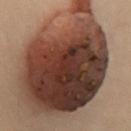Q: Was this lesion biopsied?
A: total-body-photography surveillance lesion; no biopsy
Q: What lighting was used for the tile?
A: cross-polarized
Q: Where on the body is the lesion?
A: the abdomen
Q: Automated lesion metrics?
A: an average lesion color of about L≈32 a*≈17 b*≈22 (CIELAB), about 19 CIELAB-L* units darker than the surrounding skin, and a normalized lesion–skin contrast near 16; a border-irregularity rating of about 3.5/10, a color-variation rating of about 9/10, and a peripheral color-asymmetry measure near 2.5
Q: What kind of image is this?
A: total-body-photography crop, ~15 mm field of view
Q: What is the lesion's diameter?
A: ≈15 mm
Q: Patient demographics?
A: female, aged approximately 60